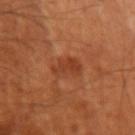From the arm. A male patient aged approximately 50. Approximately 3 mm at its widest. A 15 mm crop from a total-body photograph taken for skin-cancer surveillance.